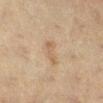Notes:
- location — the right lower leg
- acquisition — ~15 mm tile from a whole-body skin photo
- lesion diameter — ≈3 mm
- lighting — cross-polarized
- image-analysis metrics — an average lesion color of about L≈48 a*≈13 b*≈28 (CIELAB), about 6 CIELAB-L* units darker than the surrounding skin, and a normalized border contrast of about 5.5; a border-irregularity rating of about 4.5/10 and a within-lesion color-variation index near 1/10; a classifier nevus-likeness of about 0/100 and a detector confidence of about 100 out of 100 that the crop contains a lesion
- subject — female, aged approximately 55